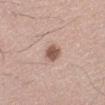Q: Is there a histopathology result?
A: catalogued during a skin exam; not biopsied
Q: Where on the body is the lesion?
A: the left lower leg
Q: Lesion size?
A: ~2.5 mm (longest diameter)
Q: What is the imaging modality?
A: total-body-photography crop, ~15 mm field of view
Q: Illumination type?
A: white-light
Q: Who is the patient?
A: male, about 35 years old